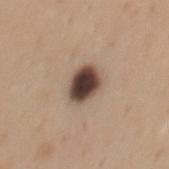Part of a total-body skin-imaging series; this lesion was reviewed on a skin check and was not flagged for biopsy. The lesion-visualizer software estimated a footprint of about 8.5 mm² and an outline eccentricity of about 0.65 (0 = round, 1 = elongated). The analysis additionally found an average lesion color of about L≈42 a*≈15 b*≈23 (CIELAB), a lesion–skin lightness drop of about 22, and a normalized border contrast of about 15.5. A male subject, aged 33–37. The lesion's longest dimension is about 4 mm. Located on the mid back. A 15 mm crop from a total-body photograph taken for skin-cancer surveillance. The tile uses white-light illumination.